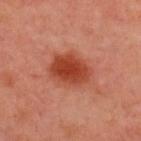Q: Was a biopsy performed?
A: catalogued during a skin exam; not biopsied
Q: Lesion location?
A: the upper back
Q: What kind of image is this?
A: 15 mm crop, total-body photography
Q: Patient demographics?
A: male, in their 50s